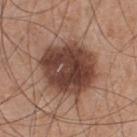follow-up: no biopsy performed (imaged during a skin exam) | subject: male, aged 53 to 57 | image source: ~15 mm tile from a whole-body skin photo | automated metrics: a footprint of about 31 mm² and an outline eccentricity of about 0.45 (0 = round, 1 = elongated); a lesion color around L≈41 a*≈20 b*≈25 in CIELAB, about 16 CIELAB-L* units darker than the surrounding skin, and a normalized border contrast of about 12 | illumination: white-light illumination | location: the chest.